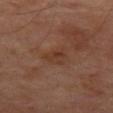Notes:
* workup · no biopsy performed (imaged during a skin exam)
* automated lesion analysis · border irregularity of about 4 on a 0–10 scale, a within-lesion color-variation index near 3/10, and a peripheral color-asymmetry measure near 1; a classifier nevus-likeness of about 0/100
* location · the left thigh
* patient · male, aged 68 to 72
* image · 15 mm crop, total-body photography